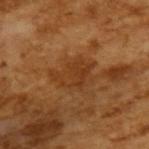notes: imaged on a skin check; not biopsied | subject: male, roughly 65 years of age | image: ~15 mm crop, total-body skin-cancer survey | diameter: ≈5 mm.